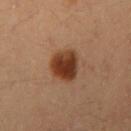This lesion was catalogued during total-body skin photography and was not selected for biopsy. A female subject approximately 35 years of age. The lesion-visualizer software estimated a symmetry-axis asymmetry near 0.2. It also reported a lesion color around L≈38 a*≈23 b*≈32 in CIELAB and a lesion–skin lightness drop of about 15. The software also gave a border-irregularity index near 2/10, a within-lesion color-variation index near 5/10, and a peripheral color-asymmetry measure near 1.5. The analysis additionally found a classifier nevus-likeness of about 100/100 and a detector confidence of about 100 out of 100 that the crop contains a lesion. From the right upper arm. A close-up tile cropped from a whole-body skin photograph, about 15 mm across.